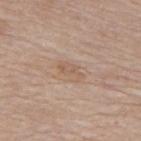Assessment:
Recorded during total-body skin imaging; not selected for excision or biopsy.
Background:
Measured at roughly 3.5 mm in maximum diameter. Cropped from a whole-body photographic skin survey; the tile spans about 15 mm. A male patient, about 75 years old. Captured under white-light illumination. An algorithmic analysis of the crop reported an area of roughly 3.5 mm² and a shape-asymmetry score of about 0.35 (0 = symmetric). The analysis additionally found a lesion color around L≈58 a*≈16 b*≈29 in CIELAB, a lesion–skin lightness drop of about 7, and a normalized border contrast of about 5. The software also gave an automated nevus-likeness rating near 0 out of 100 and a lesion-detection confidence of about 100/100. From the upper back.